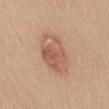biopsy status: imaged on a skin check; not biopsied | imaging modality: ~15 mm tile from a whole-body skin photo | body site: the mid back | subject: female, about 35 years old | lighting: white-light.